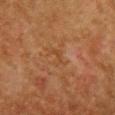illumination = cross-polarized
size = ≈2.5 mm
site = the chest
subject = female, approximately 50 years of age
image source = ~15 mm tile from a whole-body skin photo